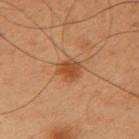Q: Is there a histopathology result?
A: imaged on a skin check; not biopsied
Q: What kind of image is this?
A: ~15 mm crop, total-body skin-cancer survey
Q: Illumination type?
A: cross-polarized illumination
Q: What did automated image analysis measure?
A: a lesion area of about 5 mm², an outline eccentricity of about 0.45 (0 = round, 1 = elongated), and two-axis asymmetry of about 0.2; a lesion color around L≈39 a*≈21 b*≈32 in CIELAB and a normalized lesion–skin contrast near 7.5; a color-variation rating of about 2.5/10 and peripheral color asymmetry of about 1
Q: What are the patient's age and sex?
A: male, aged approximately 55
Q: Lesion size?
A: about 2.5 mm
Q: What is the anatomic site?
A: the right upper arm